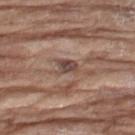Assessment:
The lesion was tiled from a total-body skin photograph and was not biopsied.
Acquisition and patient details:
A lesion tile, about 15 mm wide, cut from a 3D total-body photograph. A female subject aged 73 to 77. On the left thigh.